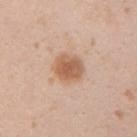Q: Was a biopsy performed?
A: imaged on a skin check; not biopsied
Q: What is the imaging modality?
A: 15 mm crop, total-body photography
Q: Patient demographics?
A: female, about 20 years old
Q: Lesion location?
A: the arm
Q: What lighting was used for the tile?
A: white-light